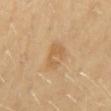<tbp_lesion>
  <automated_metrics>
    <area_mm2_approx>6.5</area_mm2_approx>
    <shape_asymmetry>0.35</shape_asymmetry>
    <cielab_L>61</cielab_L>
    <cielab_a>18</cielab_a>
    <cielab_b>40</cielab_b>
    <vs_skin_darker_L>7.0</vs_skin_darker_L>
    <vs_skin_contrast_norm>5.5</vs_skin_contrast_norm>
  </automated_metrics>
  <patient>
    <sex>female</sex>
    <age_approx>70</age_approx>
  </patient>
  <lesion_size>
    <long_diameter_mm_approx>3.5</long_diameter_mm_approx>
  </lesion_size>
  <lighting>cross-polarized</lighting>
  <site>mid back</site>
  <image>
    <source>total-body photography crop</source>
    <field_of_view_mm>15</field_of_view_mm>
  </image>
</tbp_lesion>